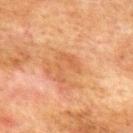Part of a total-body skin-imaging series; this lesion was reviewed on a skin check and was not flagged for biopsy. From the upper back. About 3.5 mm across. The patient is a male about 75 years old. A 15 mm close-up tile from a total-body photography series done for melanoma screening.